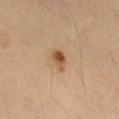Impression:
Part of a total-body skin-imaging series; this lesion was reviewed on a skin check and was not flagged for biopsy.
Clinical summary:
A male subject, aged 38–42. A 15 mm close-up extracted from a 3D total-body photography capture. From the left thigh.